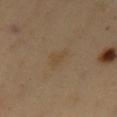This lesion was catalogued during total-body skin photography and was not selected for biopsy.
From the chest.
This is a cross-polarized tile.
A 15 mm crop from a total-body photograph taken for skin-cancer surveillance.
The patient is a male about 50 years old.
About 3 mm across.